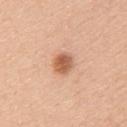{"biopsy_status": "not biopsied; imaged during a skin examination", "lighting": "white-light", "image": {"source": "total-body photography crop", "field_of_view_mm": 15}, "site": "upper back", "lesion_size": {"long_diameter_mm_approx": 3.0}, "patient": {"sex": "female", "age_approx": 40}}